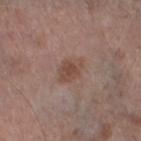workup: imaged on a skin check; not biopsied | subject: male, aged approximately 75 | imaging modality: ~15 mm crop, total-body skin-cancer survey | lesion size: about 4 mm | image-analysis metrics: a lesion area of about 5.5 mm² and a shape eccentricity near 0.8; an average lesion color of about L≈47 a*≈19 b*≈24 (CIELAB) and roughly 8 lightness units darker than nearby skin; a border-irregularity index near 2.5/10, a color-variation rating of about 2/10, and a peripheral color-asymmetry measure near 0.5; an automated nevus-likeness rating near 5 out of 100 | illumination: white-light | body site: the left thigh.